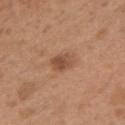No biopsy was performed on this lesion — it was imaged during a full skin examination and was not determined to be concerning.
Cropped from a whole-body photographic skin survey; the tile spans about 15 mm.
From the arm.
The lesion's longest dimension is about 3 mm.
Imaged with white-light lighting.
A female patient, approximately 30 years of age.